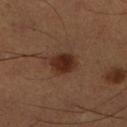Impression:
Part of a total-body skin-imaging series; this lesion was reviewed on a skin check and was not flagged for biopsy.